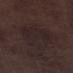Impression:
The lesion was tiled from a total-body skin photograph and was not biopsied.
Clinical summary:
A male subject, aged 68 to 72. Located on the left lower leg. Cropped from a whole-body photographic skin survey; the tile spans about 15 mm.